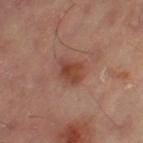Findings:
– biopsy status · total-body-photography surveillance lesion; no biopsy
– image-analysis metrics · a shape eccentricity near 0.45; a classifier nevus-likeness of about 55/100 and a lesion-detection confidence of about 100/100
– image · 15 mm crop, total-body photography
– size · ≈3 mm
– body site · the left thigh